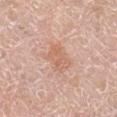Case summary:
- biopsy status: imaged on a skin check; not biopsied
- patient: female, roughly 55 years of age
- illumination: white-light illumination
- body site: the right lower leg
- lesion size: about 3.5 mm
- imaging modality: ~15 mm tile from a whole-body skin photo